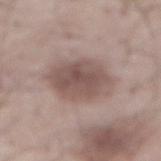Context: A close-up tile cropped from a whole-body skin photograph, about 15 mm across. A male subject, about 55 years old. The lesion's longest dimension is about 6 mm. On the abdomen.